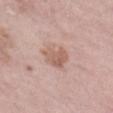  biopsy_status: not biopsied; imaged during a skin examination
  image:
    source: total-body photography crop
    field_of_view_mm: 15
  site: right lower leg
  lesion_size:
    long_diameter_mm_approx: 4.0
  patient:
    sex: female
    age_approx: 70
  automated_metrics:
    cielab_L: 62
    cielab_a: 19
    cielab_b: 26
    vs_skin_darker_L: 8.0
    border_irregularity_0_10: 2.0
    peripheral_color_asymmetry: 1.5
    nevus_likeness_0_100: 0
  lighting: white-light